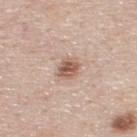This lesion was catalogued during total-body skin photography and was not selected for biopsy.
A male subject in their mid-40s.
The lesion-visualizer software estimated a footprint of about 5.5 mm², an outline eccentricity of about 0.5 (0 = round, 1 = elongated), and two-axis asymmetry of about 0.15. It also reported a normalized lesion–skin contrast near 8.5.
This is a white-light tile.
The recorded lesion diameter is about 2.5 mm.
The lesion is located on the upper back.
A 15 mm close-up extracted from a 3D total-body photography capture.